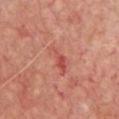A 15 mm crop from a total-body photograph taken for skin-cancer surveillance. A subject roughly 55 years of age. From the chest.